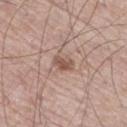The lesion was tiled from a total-body skin photograph and was not biopsied.
A male subject in their 70s.
Measured at roughly 3 mm in maximum diameter.
On the leg.
Cropped from a whole-body photographic skin survey; the tile spans about 15 mm.